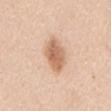Assessment:
Part of a total-body skin-imaging series; this lesion was reviewed on a skin check and was not flagged for biopsy.
Context:
On the mid back. A male patient aged approximately 60. Captured under white-light illumination. An algorithmic analysis of the crop reported a footprint of about 10 mm². The analysis additionally found a lesion color around L≈65 a*≈20 b*≈34 in CIELAB, a lesion–skin lightness drop of about 13, and a normalized border contrast of about 8. And it measured an automated nevus-likeness rating near 95 out of 100 and a detector confidence of about 100 out of 100 that the crop contains a lesion. Cropped from a whole-body photographic skin survey; the tile spans about 15 mm. Approximately 5 mm at its widest.